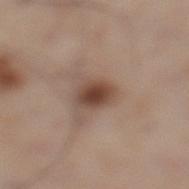Impression: This lesion was catalogued during total-body skin photography and was not selected for biopsy. Clinical summary: A male patient, aged 58–62. A roughly 15 mm field-of-view crop from a total-body skin photograph. Approximately 3.5 mm at its widest. The lesion is located on the leg.